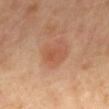Recorded during total-body skin imaging; not selected for excision or biopsy.
This is a cross-polarized tile.
The lesion-visualizer software estimated a lesion area of about 4.5 mm² and an eccentricity of roughly 0.8. The software also gave a border-irregularity index near 2/10 and a within-lesion color-variation index near 2.5/10.
This image is a 15 mm lesion crop taken from a total-body photograph.
From the mid back.